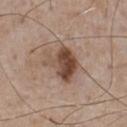follow-up = imaged on a skin check; not biopsied
anatomic site = the chest
imaging modality = total-body-photography crop, ~15 mm field of view
subject = male, approximately 75 years of age
lesion diameter = about 4.5 mm
illumination = white-light